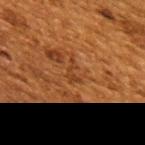| field | value |
|---|---|
| notes | total-body-photography surveillance lesion; no biopsy |
| patient | female, in their 50s |
| imaging modality | total-body-photography crop, ~15 mm field of view |
| image-analysis metrics | a footprint of about 2.5 mm² and two-axis asymmetry of about 0.6; an average lesion color of about L≈33 a*≈22 b*≈33 (CIELAB) |
| site | the upper back |